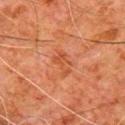{"biopsy_status": "not biopsied; imaged during a skin examination", "image": {"source": "total-body photography crop", "field_of_view_mm": 15}, "patient": {"sex": "male", "age_approx": 80}, "site": "chest"}